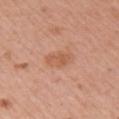The lesion was tiled from a total-body skin photograph and was not biopsied.